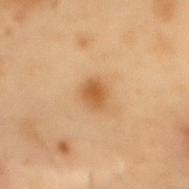Part of a total-body skin-imaging series; this lesion was reviewed on a skin check and was not flagged for biopsy. A male patient, roughly 55 years of age. Approximately 2.5 mm at its widest. This is a cross-polarized tile. A 15 mm close-up extracted from a 3D total-body photography capture. Automated tile analysis of the lesion measured an eccentricity of roughly 0.55 and a shape-asymmetry score of about 0.25 (0 = symmetric). The analysis additionally found a classifier nevus-likeness of about 95/100 and a detector confidence of about 100 out of 100 that the crop contains a lesion. The lesion is located on the mid back.